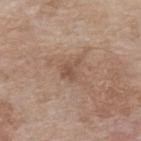Clinical summary:
From the back. The patient is a female aged around 75. Automated tile analysis of the lesion measured a detector confidence of about 100 out of 100 that the crop contains a lesion. A close-up tile cropped from a whole-body skin photograph, about 15 mm across. Longest diameter approximately 2.5 mm.